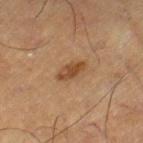Imaged during a routine full-body skin examination; the lesion was not biopsied and no histopathology is available.
The lesion is located on the right lower leg.
Automated image analysis of the tile measured a lesion area of about 4.5 mm² and a shape eccentricity near 0.85. And it measured a lesion color around L≈38 a*≈17 b*≈30 in CIELAB, a lesion–skin lightness drop of about 9, and a normalized border contrast of about 8.5. The software also gave a border-irregularity rating of about 2/10, a color-variation rating of about 3.5/10, and radial color variation of about 1. And it measured a nevus-likeness score of about 75/100 and lesion-presence confidence of about 100/100.
Measured at roughly 3.5 mm in maximum diameter.
The subject is a male about 60 years old.
A 15 mm close-up tile from a total-body photography series done for melanoma screening.